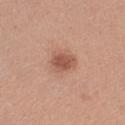The lesion was photographed on a routine skin check and not biopsied; there is no pathology result. Imaged with white-light lighting. A female subject aged approximately 55. An algorithmic analysis of the crop reported a shape eccentricity near 0.75 and a symmetry-axis asymmetry near 0.2. The software also gave a border-irregularity index near 2/10 and a color-variation rating of about 2.5/10. This image is a 15 mm lesion crop taken from a total-body photograph. About 3.5 mm across. The lesion is located on the left thigh.